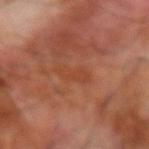Q: Is there a histopathology result?
A: total-body-photography surveillance lesion; no biopsy
Q: How was this image acquired?
A: total-body-photography crop, ~15 mm field of view
Q: How was the tile lit?
A: cross-polarized illumination
Q: Lesion location?
A: the right forearm
Q: What is the lesion's diameter?
A: ≈4.5 mm
Q: What did automated image analysis measure?
A: a footprint of about 4 mm² and a shape-asymmetry score of about 0.5 (0 = symmetric); a classifier nevus-likeness of about 0/100
Q: Patient demographics?
A: male, aged 68 to 72